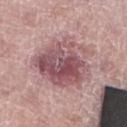Impression: No biopsy was performed on this lesion — it was imaged during a full skin examination and was not determined to be concerning. Clinical summary: The lesion's longest dimension is about 8.5 mm. From the left lower leg. A 15 mm close-up extracted from a 3D total-body photography capture. Captured under white-light illumination. The total-body-photography lesion software estimated a mean CIELAB color near L≈53 a*≈23 b*≈17, about 13 CIELAB-L* units darker than the surrounding skin, and a lesion-to-skin contrast of about 9 (normalized; higher = more distinct). The software also gave border irregularity of about 3 on a 0–10 scale, a within-lesion color-variation index near 9.5/10, and radial color variation of about 3.5. A female subject, roughly 60 years of age.